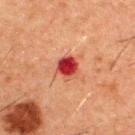notes: total-body-photography surveillance lesion; no biopsy
lesion diameter: about 3 mm
body site: the upper back
automated lesion analysis: about 15 CIELAB-L* units darker than the surrounding skin and a lesion-to-skin contrast of about 12 (normalized; higher = more distinct)
acquisition: ~15 mm crop, total-body skin-cancer survey
illumination: cross-polarized illumination
patient: male, about 50 years old